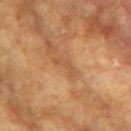Notes:
• biopsy status — no biopsy performed (imaged during a skin exam)
• imaging modality — ~15 mm crop, total-body skin-cancer survey
• lesion diameter — ~3.5 mm (longest diameter)
• patient — female, in their mid-70s
• lighting — cross-polarized
• location — the left upper arm